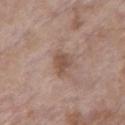patient — female, about 85 years old or older | image-analysis metrics — a shape eccentricity near 0.65 and a shape-asymmetry score of about 0.3 (0 = symmetric); a mean CIELAB color near L≈51 a*≈18 b*≈25, roughly 10 lightness units darker than nearby skin, and a lesion-to-skin contrast of about 7 (normalized; higher = more distinct); a within-lesion color-variation index near 2.5/10 and radial color variation of about 1; an automated nevus-likeness rating near 15 out of 100 | body site — the chest | illumination — white-light illumination | acquisition — total-body-photography crop, ~15 mm field of view | size — ~3 mm (longest diameter).